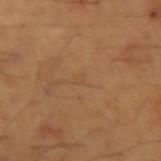The lesion was photographed on a routine skin check and not biopsied; there is no pathology result. A male subject in their mid- to late 60s. A region of skin cropped from a whole-body photographic capture, roughly 15 mm wide. Automated image analysis of the tile measured a footprint of about 1 mm², a shape eccentricity near 0.45, and two-axis asymmetry of about 0.45. It also reported border irregularity of about 4 on a 0–10 scale, a color-variation rating of about 0/10, and radial color variation of about 0. The analysis additionally found an automated nevus-likeness rating near 0 out of 100 and a detector confidence of about 95 out of 100 that the crop contains a lesion. Imaged with cross-polarized lighting. About 1.5 mm across. From the left forearm.